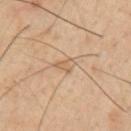The lesion was tiled from a total-body skin photograph and was not biopsied.
Automated tile analysis of the lesion measured a color-variation rating of about 1/10 and radial color variation of about 0.5.
A roughly 15 mm field-of-view crop from a total-body skin photograph.
This is a cross-polarized tile.
A male subject roughly 40 years of age.
From the left upper arm.